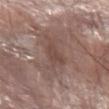Recorded during total-body skin imaging; not selected for excision or biopsy. The subject is a male aged 63 to 67. A 15 mm crop from a total-body photograph taken for skin-cancer surveillance. This is a white-light tile. The recorded lesion diameter is about 4 mm. Located on the left forearm.